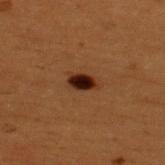biopsy status: catalogued during a skin exam; not biopsied
patient: male, aged 48–52
lighting: cross-polarized
imaging modality: total-body-photography crop, ~15 mm field of view
diameter: about 3 mm
site: the upper back